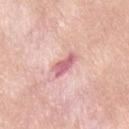{
  "biopsy_status": "not biopsied; imaged during a skin examination",
  "patient": {
    "sex": "female",
    "age_approx": 35
  },
  "site": "lower back",
  "image": {
    "source": "total-body photography crop",
    "field_of_view_mm": 15
  }
}